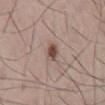This is a white-light tile. A male subject, aged 73–77. A 15 mm close-up extracted from a 3D total-body photography capture. On the mid back.